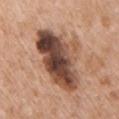workup: no biopsy performed (imaged during a skin exam)
lesion diameter: about 8.5 mm
anatomic site: the chest
subject: male, approximately 65 years of age
acquisition: total-body-photography crop, ~15 mm field of view
lighting: white-light illumination